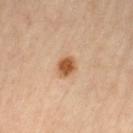The lesion is on the left thigh.
The subject is a female aged 63–67.
Cropped from a total-body skin-imaging series; the visible field is about 15 mm.